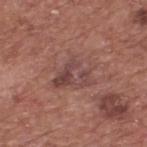Imaged during a routine full-body skin examination; the lesion was not biopsied and no histopathology is available. The patient is a male about 75 years old. Imaged with white-light lighting. A 15 mm close-up extracted from a 3D total-body photography capture. The lesion is on the back.